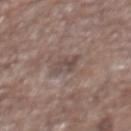anatomic site: the abdomen
image: total-body-photography crop, ~15 mm field of view
subject: male, approximately 70 years of age
illumination: white-light